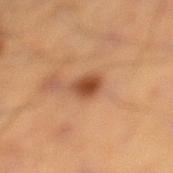Captured during whole-body skin photography for melanoma surveillance; the lesion was not biopsied. A male subject, aged around 55. The tile uses cross-polarized illumination. Automated image analysis of the tile measured a lesion color around L≈41 a*≈22 b*≈31 in CIELAB and roughly 12 lightness units darker than nearby skin. It also reported border irregularity of about 2.5 on a 0–10 scale and a within-lesion color-variation index near 3/10. From the right lower leg. Cropped from a whole-body photographic skin survey; the tile spans about 15 mm.